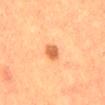<case>
<biopsy_status>not biopsied; imaged during a skin examination</biopsy_status>
<lighting>cross-polarized</lighting>
<automated_metrics>
  <area_mm2_approx>3.5</area_mm2_approx>
  <eccentricity>0.75</eccentricity>
  <shape_asymmetry>0.2</shape_asymmetry>
  <nevus_likeness_0_100>90</nevus_likeness_0_100>
  <lesion_detection_confidence_0_100>100</lesion_detection_confidence_0_100>
</automated_metrics>
<lesion_size>
  <long_diameter_mm_approx>2.5</long_diameter_mm_approx>
</lesion_size>
<site>mid back</site>
<patient>
  <sex>male</sex>
  <age_approx>50</age_approx>
</patient>
<image>
  <source>total-body photography crop</source>
  <field_of_view_mm>15</field_of_view_mm>
</image>
</case>